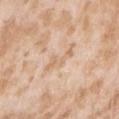Case summary:
– workup · imaged on a skin check; not biopsied